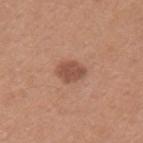Assessment:
No biopsy was performed on this lesion — it was imaged during a full skin examination and was not determined to be concerning.
Image and clinical context:
Approximately 3.5 mm at its widest. On the arm. The tile uses white-light illumination. A male subject, approximately 30 years of age. A lesion tile, about 15 mm wide, cut from a 3D total-body photograph. Automated image analysis of the tile measured a lesion area of about 6 mm², an outline eccentricity of about 0.7 (0 = round, 1 = elongated), and two-axis asymmetry of about 0.2. It also reported a nevus-likeness score of about 70/100 and a lesion-detection confidence of about 100/100.